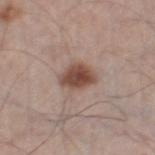Recorded during total-body skin imaging; not selected for excision or biopsy.
This image is a 15 mm lesion crop taken from a total-body photograph.
Captured under white-light illumination.
About 3.5 mm across.
The lesion-visualizer software estimated a lesion area of about 7.5 mm², a shape eccentricity near 0.7, and a symmetry-axis asymmetry near 0.25.
A male patient, aged around 60.
The lesion is on the right thigh.